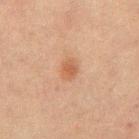Impression: Part of a total-body skin-imaging series; this lesion was reviewed on a skin check and was not flagged for biopsy. Background: The lesion is located on the mid back. Captured under cross-polarized illumination. Cropped from a whole-body photographic skin survey; the tile spans about 15 mm. The total-body-photography lesion software estimated a color-variation rating of about 2/10 and a peripheral color-asymmetry measure near 0.5. The lesion's longest dimension is about 2.5 mm. A male patient aged 63–67.